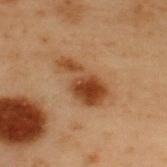| field | value |
|---|---|
| follow-up | catalogued during a skin exam; not biopsied |
| lesion diameter | about 6 mm |
| patient | male, aged 53 to 57 |
| site | the upper back |
| imaging modality | total-body-photography crop, ~15 mm field of view |